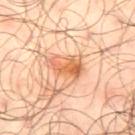* follow-up: imaged on a skin check; not biopsied
* patient: male, about 65 years old
* acquisition: 15 mm crop, total-body photography
* lesion diameter: ≈4.5 mm
* site: the right thigh